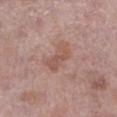Impression:
This lesion was catalogued during total-body skin photography and was not selected for biopsy.
Image and clinical context:
A female patient in their 70s. A 15 mm close-up extracted from a 3D total-body photography capture. On the left lower leg. Longest diameter approximately 3.5 mm. An algorithmic analysis of the crop reported a shape eccentricity near 0.9 and a shape-asymmetry score of about 0.25 (0 = symmetric). It also reported an average lesion color of about L≈53 a*≈21 b*≈26 (CIELAB), about 8 CIELAB-L* units darker than the surrounding skin, and a normalized lesion–skin contrast near 6.